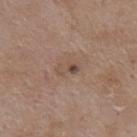follow-up: imaged on a skin check; not biopsied
image source: total-body-photography crop, ~15 mm field of view
TBP lesion metrics: a lesion area of about 4.5 mm², a shape eccentricity near 0.85, and two-axis asymmetry of about 0.25
lighting: white-light illumination
site: the back
subject: female, about 70 years old
size: ~3 mm (longest diameter)